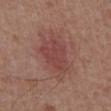follow-up = catalogued during a skin exam; not biopsied | tile lighting = white-light | lesion diameter = about 7 mm | patient = male, in their mid- to late 50s | body site = the front of the torso | image source = ~15 mm tile from a whole-body skin photo.